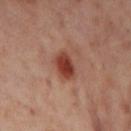Imaged during a routine full-body skin examination; the lesion was not biopsied and no histopathology is available.
A close-up tile cropped from a whole-body skin photograph, about 15 mm across.
A female patient, aged 53–57.
Measured at roughly 3.5 mm in maximum diameter.
From the left thigh.